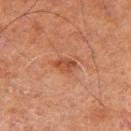{"biopsy_status": "not biopsied; imaged during a skin examination", "lighting": "cross-polarized", "site": "arm", "automated_metrics": {"area_mm2_approx": 4.0, "eccentricity": 0.8, "shape_asymmetry": 0.25, "border_irregularity_0_10": 2.0, "color_variation_0_10": 2.5, "peripheral_color_asymmetry": 1.0}, "image": {"source": "total-body photography crop", "field_of_view_mm": 15}, "patient": {"sex": "male", "age_approx": 70}}